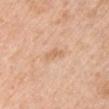The lesion was photographed on a routine skin check and not biopsied; there is no pathology result.
The lesion's longest dimension is about 2.5 mm.
A female subject, in their mid- to late 40s.
From the right upper arm.
The tile uses white-light illumination.
This image is a 15 mm lesion crop taken from a total-body photograph.